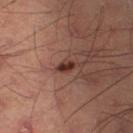Recorded during total-body skin imaging; not selected for excision or biopsy. This image is a 15 mm lesion crop taken from a total-body photograph. The recorded lesion diameter is about 2 mm. Located on the left thigh. Imaged with cross-polarized lighting. The subject is a male aged 48–52. Automated tile analysis of the lesion measured a footprint of about 2 mm², a shape eccentricity near 0.8, and a shape-asymmetry score of about 0.35 (0 = symmetric). And it measured a lesion-to-skin contrast of about 12.5 (normalized; higher = more distinct). The software also gave border irregularity of about 3 on a 0–10 scale, internal color variation of about 0 on a 0–10 scale, and a peripheral color-asymmetry measure near 0.